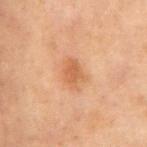Captured during whole-body skin photography for melanoma surveillance; the lesion was not biopsied. From the chest. Cropped from a total-body skin-imaging series; the visible field is about 15 mm. The subject is a male approximately 70 years of age. About 3.5 mm across. The lesion-visualizer software estimated a footprint of about 6 mm², a shape eccentricity near 0.7, and two-axis asymmetry of about 0.2. And it measured an average lesion color of about L≈46 a*≈19 b*≈31 (CIELAB). It also reported a detector confidence of about 100 out of 100 that the crop contains a lesion.